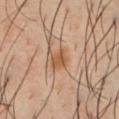biopsy_status: not biopsied; imaged during a skin examination
automated_metrics:
  cielab_L: 53
  cielab_a: 21
  cielab_b: 35
  vs_skin_contrast_norm: 8.0
  peripheral_color_asymmetry: 1.0
image:
  source: total-body photography crop
  field_of_view_mm: 15
patient:
  sex: male
  age_approx: 40
site: front of the torso
lighting: cross-polarized